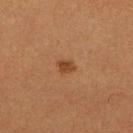<record>
  <biopsy_status>not biopsied; imaged during a skin examination</biopsy_status>
  <site>right lower leg</site>
  <lighting>cross-polarized</lighting>
  <image>
    <source>total-body photography crop</source>
    <field_of_view_mm>15</field_of_view_mm>
  </image>
  <patient>
    <sex>female</sex>
    <age_approx>30</age_approx>
  </patient>
</record>